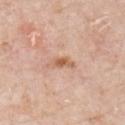| feature | finding |
|---|---|
| follow-up | imaged on a skin check; not biopsied |
| size | ~3 mm (longest diameter) |
| subject | male, aged 78 to 82 |
| image source | ~15 mm tile from a whole-body skin photo |
| lighting | white-light illumination |
| anatomic site | the chest |
| automated lesion analysis | a shape eccentricity near 0.9 and a shape-asymmetry score of about 0.4 (0 = symmetric); an average lesion color of about L≈61 a*≈22 b*≈34 (CIELAB) and about 11 CIELAB-L* units darker than the surrounding skin; a border-irregularity index near 4.5/10 and a peripheral color-asymmetry measure near 0; a nevus-likeness score of about 60/100 and a lesion-detection confidence of about 100/100 |